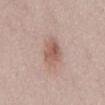workup: total-body-photography surveillance lesion; no biopsy | automated lesion analysis: a mean CIELAB color near L≈57 a*≈20 b*≈25 and a lesion-to-skin contrast of about 7 (normalized; higher = more distinct); a border-irregularity index near 2.5/10, internal color variation of about 4.5 on a 0–10 scale, and peripheral color asymmetry of about 1.5; an automated nevus-likeness rating near 70 out of 100 | patient: female, in their 50s | tile lighting: white-light | image source: 15 mm crop, total-body photography | lesion size: ≈4 mm | body site: the back.